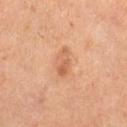Imaged during a routine full-body skin examination; the lesion was not biopsied and no histopathology is available. The lesion is on the left thigh. A female patient, aged approximately 55. The tile uses cross-polarized illumination. A region of skin cropped from a whole-body photographic capture, roughly 15 mm wide. Automated image analysis of the tile measured a border-irregularity rating of about 2/10 and internal color variation of about 5 on a 0–10 scale. And it measured a lesion-detection confidence of about 100/100.